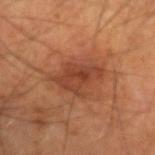follow-up: total-body-photography surveillance lesion; no biopsy
TBP lesion metrics: an average lesion color of about L≈41 a*≈25 b*≈31 (CIELAB) and about 9 CIELAB-L* units darker than the surrounding skin; border irregularity of about 4 on a 0–10 scale and internal color variation of about 3.5 on a 0–10 scale; an automated nevus-likeness rating near 5 out of 100 and a lesion-detection confidence of about 100/100
site: the arm
tile lighting: cross-polarized
lesion size: ~6 mm (longest diameter)
patient: male, aged 48 to 52
image source: 15 mm crop, total-body photography